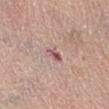This lesion was catalogued during total-body skin photography and was not selected for biopsy.
A female subject approximately 65 years of age.
The lesion-visualizer software estimated a footprint of about 3.5 mm², an outline eccentricity of about 0.75 (0 = round, 1 = elongated), and two-axis asymmetry of about 0.3. It also reported an average lesion color of about L≈56 a*≈23 b*≈18 (CIELAB), about 10 CIELAB-L* units darker than the surrounding skin, and a normalized border contrast of about 7.5. The analysis additionally found a border-irregularity rating of about 3/10. The analysis additionally found a nevus-likeness score of about 0/100.
A lesion tile, about 15 mm wide, cut from a 3D total-body photograph.
The lesion is on the abdomen.
Imaged with white-light lighting.